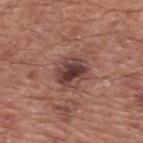The lesion was photographed on a routine skin check and not biopsied; there is no pathology result.
The lesion-visualizer software estimated a border-irregularity rating of about 2.5/10 and radial color variation of about 2.5.
A roughly 15 mm field-of-view crop from a total-body skin photograph.
This is a white-light tile.
A male subject in their mid- to late 70s.
From the back.